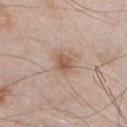| key | value |
|---|---|
| workup | no biopsy performed (imaged during a skin exam) |
| lighting | white-light |
| anatomic site | the chest |
| patient | male, in their mid- to late 50s |
| acquisition | ~15 mm tile from a whole-body skin photo |
| lesion size | ≈2.5 mm |